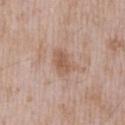– follow-up · catalogued during a skin exam; not biopsied
– site · the chest
– patient · male, aged approximately 50
– illumination · white-light
– lesion size · ~3 mm (longest diameter)
– image source · ~15 mm crop, total-body skin-cancer survey